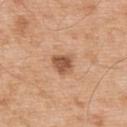biopsy status: total-body-photography surveillance lesion; no biopsy
size: about 2.5 mm
imaging modality: ~15 mm crop, total-body skin-cancer survey
body site: the upper back
subject: male, in their mid-50s